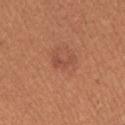The lesion was photographed on a routine skin check and not biopsied; there is no pathology result. A female patient, about 40 years old. A 15 mm close-up extracted from a 3D total-body photography capture. From the right upper arm.